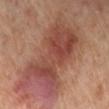Recorded during total-body skin imaging; not selected for excision or biopsy. The recorded lesion diameter is about 9.5 mm. A female patient about 55 years old. Captured under cross-polarized illumination. The lesion is located on the leg. A region of skin cropped from a whole-body photographic capture, roughly 15 mm wide.